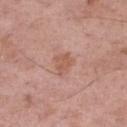Clinical impression:
Imaged during a routine full-body skin examination; the lesion was not biopsied and no histopathology is available.
Clinical summary:
The recorded lesion diameter is about 3 mm. Automated image analysis of the tile measured a footprint of about 5 mm² and a shape-asymmetry score of about 0.3 (0 = symmetric). And it measured border irregularity of about 3 on a 0–10 scale, a color-variation rating of about 1.5/10, and a peripheral color-asymmetry measure near 0.5. A female subject, in their 60s. From the right thigh. Captured under white-light illumination. A close-up tile cropped from a whole-body skin photograph, about 15 mm across.